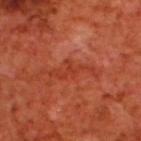Q: Is there a histopathology result?
A: no biopsy performed (imaged during a skin exam)
Q: What kind of image is this?
A: ~15 mm crop, total-body skin-cancer survey
Q: Illumination type?
A: cross-polarized illumination
Q: How large is the lesion?
A: about 5.5 mm
Q: What did automated image analysis measure?
A: an area of roughly 6 mm²
Q: Where on the body is the lesion?
A: the upper back
Q: Who is the patient?
A: male, aged approximately 70